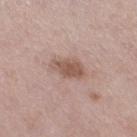Part of a total-body skin-imaging series; this lesion was reviewed on a skin check and was not flagged for biopsy.
A female patient aged approximately 40.
The lesion is located on the right thigh.
Approximately 3.5 mm at its widest.
This is a white-light tile.
A roughly 15 mm field-of-view crop from a total-body skin photograph.
The lesion-visualizer software estimated an eccentricity of roughly 0.8 and a symmetry-axis asymmetry near 0.25.